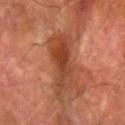Captured during whole-body skin photography for melanoma surveillance; the lesion was not biopsied.
The lesion is on the left forearm.
A male patient, about 80 years old.
A 15 mm close-up tile from a total-body photography series done for melanoma screening.
This is a cross-polarized tile.